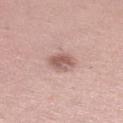location: the left leg; tile lighting: white-light illumination; diameter: ≈3 mm; patient: female, aged approximately 40; imaging modality: total-body-photography crop, ~15 mm field of view.